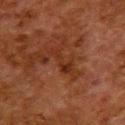Captured during whole-body skin photography for melanoma surveillance; the lesion was not biopsied. The total-body-photography lesion software estimated a border-irregularity rating of about 6.5/10, internal color variation of about 2 on a 0–10 scale, and peripheral color asymmetry of about 0.5. The software also gave a classifier nevus-likeness of about 0/100 and lesion-presence confidence of about 100/100. Located on the upper back. A roughly 15 mm field-of-view crop from a total-body skin photograph. The tile uses cross-polarized illumination. The recorded lesion diameter is about 4 mm. A female subject aged 48 to 52.